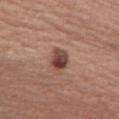biopsy status: no biopsy performed (imaged during a skin exam); patient: female, aged 63 to 67; body site: the chest; tile lighting: white-light; image: ~15 mm tile from a whole-body skin photo.